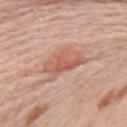follow-up: imaged on a skin check; not biopsied
imaging modality: ~15 mm crop, total-body skin-cancer survey
lighting: white-light
image-analysis metrics: a lesion area of about 10 mm², an eccentricity of roughly 0.8, and a shape-asymmetry score of about 0.35 (0 = symmetric); border irregularity of about 4.5 on a 0–10 scale and a within-lesion color-variation index near 3.5/10; a classifier nevus-likeness of about 5/100 and a lesion-detection confidence of about 100/100
body site: the right upper arm
subject: female, about 65 years old